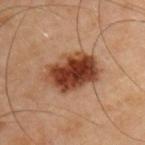The lesion was photographed on a routine skin check and not biopsied; there is no pathology result.
The recorded lesion diameter is about 6.5 mm.
A male patient, aged around 45.
Captured under cross-polarized illumination.
Cropped from a whole-body photographic skin survey; the tile spans about 15 mm.
The lesion is located on the left upper arm.
The lesion-visualizer software estimated an area of roughly 21 mm², an outline eccentricity of about 0.75 (0 = round, 1 = elongated), and two-axis asymmetry of about 0.15. The software also gave internal color variation of about 8.5 on a 0–10 scale and a peripheral color-asymmetry measure near 3. The software also gave a lesion-detection confidence of about 100/100.